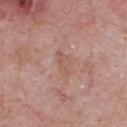The lesion was tiled from a total-body skin photograph and was not biopsied. A male subject aged around 55. The recorded lesion diameter is about 3.5 mm. Imaged with white-light lighting. A close-up tile cropped from a whole-body skin photograph, about 15 mm across. On the front of the torso.